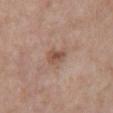<tbp_lesion>
  <biopsy_status>not biopsied; imaged during a skin examination</biopsy_status>
  <lesion_size>
    <long_diameter_mm_approx>2.5</long_diameter_mm_approx>
  </lesion_size>
  <image>
    <source>total-body photography crop</source>
    <field_of_view_mm>15</field_of_view_mm>
  </image>
  <automated_metrics>
    <area_mm2_approx>4.0</area_mm2_approx>
    <eccentricity>0.6</eccentricity>
    <cielab_L>51</cielab_L>
    <cielab_a>19</cielab_a>
    <cielab_b>28</cielab_b>
    <vs_skin_darker_L>9.0</vs_skin_darker_L>
    <vs_skin_contrast_norm>7.0</vs_skin_contrast_norm>
    <border_irregularity_0_10>3.0</border_irregularity_0_10>
    <color_variation_0_10>3.5</color_variation_0_10>
    <peripheral_color_asymmetry>1.5</peripheral_color_asymmetry>
  </automated_metrics>
  <site>chest</site>
  <lighting>white-light</lighting>
  <patient>
    <sex>female</sex>
    <age_approx>55</age_approx>
  </patient>
</tbp_lesion>